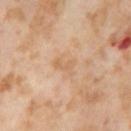{
  "biopsy_status": "not biopsied; imaged during a skin examination",
  "site": "right thigh",
  "automated_metrics": {
    "nevus_likeness_0_100": 0,
    "lesion_detection_confidence_0_100": 100
  },
  "patient": {
    "sex": "female",
    "age_approx": 55
  },
  "lighting": "cross-polarized",
  "image": {
    "source": "total-body photography crop",
    "field_of_view_mm": 15
  },
  "lesion_size": {
    "long_diameter_mm_approx": 2.5
  }
}